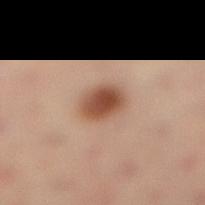notes: total-body-photography surveillance lesion; no biopsy | size: about 3.5 mm | patient: female, aged 33–37 | illumination: cross-polarized illumination | imaging modality: 15 mm crop, total-body photography | body site: the leg.